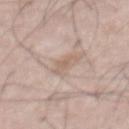Clinical impression:
This lesion was catalogued during total-body skin photography and was not selected for biopsy.
Clinical summary:
An algorithmic analysis of the crop reported a footprint of about 3 mm² and two-axis asymmetry of about 0.45. The software also gave a lesion–skin lightness drop of about 8 and a normalized lesion–skin contrast near 5.5. The software also gave internal color variation of about 0.5 on a 0–10 scale. And it measured a classifier nevus-likeness of about 0/100 and a lesion-detection confidence of about 60/100. The lesion's longest dimension is about 3 mm. A lesion tile, about 15 mm wide, cut from a 3D total-body photograph. A male patient approximately 55 years of age. Located on the mid back. Captured under white-light illumination.